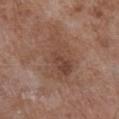This lesion was catalogued during total-body skin photography and was not selected for biopsy.
From the chest.
A region of skin cropped from a whole-body photographic capture, roughly 15 mm wide.
The lesion's longest dimension is about 6.5 mm.
A female subject approximately 80 years of age.